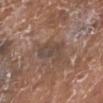Assessment:
The lesion was tiled from a total-body skin photograph and was not biopsied.
Context:
This is a white-light tile. Measured at roughly 5 mm in maximum diameter. A female subject, aged 73–77. On the left lower leg. The total-body-photography lesion software estimated an area of roughly 10 mm², an outline eccentricity of about 0.85 (0 = round, 1 = elongated), and a symmetry-axis asymmetry near 0.3. It also reported border irregularity of about 3.5 on a 0–10 scale, a color-variation rating of about 5/10, and radial color variation of about 2. The software also gave lesion-presence confidence of about 65/100. A 15 mm crop from a total-body photograph taken for skin-cancer surveillance.